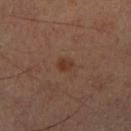<record>
<biopsy_status>not biopsied; imaged during a skin examination</biopsy_status>
<patient>
  <sex>male</sex>
  <age_approx>60</age_approx>
</patient>
<lesion_size>
  <long_diameter_mm_approx>2.0</long_diameter_mm_approx>
</lesion_size>
<site>leg</site>
<lighting>cross-polarized</lighting>
<image>
  <source>total-body photography crop</source>
  <field_of_view_mm>15</field_of_view_mm>
</image>
</record>